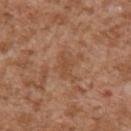Q: Was a biopsy performed?
A: imaged on a skin check; not biopsied
Q: Lesion location?
A: the right upper arm
Q: What kind of image is this?
A: ~15 mm tile from a whole-body skin photo
Q: How large is the lesion?
A: about 3 mm
Q: What are the patient's age and sex?
A: male, aged 43–47
Q: What did automated image analysis measure?
A: a lesion area of about 3.5 mm², an eccentricity of roughly 0.8, and a symmetry-axis asymmetry near 0.5; an average lesion color of about L≈48 a*≈21 b*≈33 (CIELAB), a lesion–skin lightness drop of about 6, and a normalized lesion–skin contrast near 5; internal color variation of about 0.5 on a 0–10 scale and a peripheral color-asymmetry measure near 0.5; an automated nevus-likeness rating near 0 out of 100 and a detector confidence of about 95 out of 100 that the crop contains a lesion